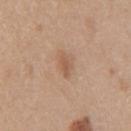| feature | finding |
|---|---|
| biopsy status | total-body-photography surveillance lesion; no biopsy |
| acquisition | total-body-photography crop, ~15 mm field of view |
| subject | female, roughly 40 years of age |
| automated lesion analysis | a footprint of about 3.5 mm² and a symmetry-axis asymmetry near 0.3 |
| site | the right upper arm |
| illumination | white-light illumination |
| lesion diameter | ~2.5 mm (longest diameter) |